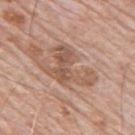Imaged during a routine full-body skin examination; the lesion was not biopsied and no histopathology is available.
Imaged with white-light lighting.
Located on the mid back.
The recorded lesion diameter is about 6 mm.
A male patient in their 80s.
Automated tile analysis of the lesion measured border irregularity of about 10 on a 0–10 scale and radial color variation of about 1. And it measured lesion-presence confidence of about 100/100.
A 15 mm close-up extracted from a 3D total-body photography capture.